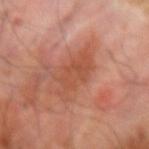Q: Is there a histopathology result?
A: catalogued during a skin exam; not biopsied
Q: How large is the lesion?
A: about 5 mm
Q: What kind of image is this?
A: ~15 mm crop, total-body skin-cancer survey
Q: Lesion location?
A: the right forearm
Q: Patient demographics?
A: male, aged 68 to 72